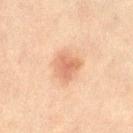Imaged during a routine full-body skin examination; the lesion was not biopsied and no histopathology is available. A female patient, aged approximately 45. The total-body-photography lesion software estimated an area of roughly 7.5 mm², a shape eccentricity near 0.5, and a shape-asymmetry score of about 0.35 (0 = symmetric). The analysis additionally found a mean CIELAB color near L≈59 a*≈21 b*≈31, about 9 CIELAB-L* units darker than the surrounding skin, and a lesion-to-skin contrast of about 6.5 (normalized; higher = more distinct). The software also gave border irregularity of about 3 on a 0–10 scale, a color-variation rating of about 3.5/10, and peripheral color asymmetry of about 1.5. About 3.5 mm across. Imaged with cross-polarized lighting. The lesion is located on the right thigh. Cropped from a total-body skin-imaging series; the visible field is about 15 mm.